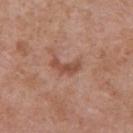The lesion was photographed on a routine skin check and not biopsied; there is no pathology result. About 3.5 mm across. This image is a 15 mm lesion crop taken from a total-body photograph. The patient is a female aged approximately 60. The lesion is located on the upper back.